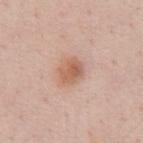A 15 mm close-up extracted from a 3D total-body photography capture.
The lesion's longest dimension is about 3 mm.
The tile uses white-light illumination.
A male patient aged around 55.
Located on the abdomen.